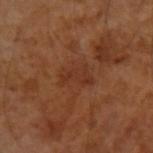follow-up — imaged on a skin check; not biopsied
location — the left forearm
image — ~15 mm tile from a whole-body skin photo
patient — male, aged 53–57